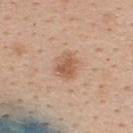{
  "biopsy_status": "not biopsied; imaged during a skin examination",
  "image": {
    "source": "total-body photography crop",
    "field_of_view_mm": 15
  },
  "site": "upper back",
  "patient": {
    "sex": "female",
    "age_approx": 40
  }
}